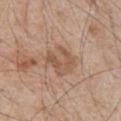No biopsy was performed on this lesion — it was imaged during a full skin examination and was not determined to be concerning. Captured under white-light illumination. The lesion-visualizer software estimated a lesion-to-skin contrast of about 6 (normalized; higher = more distinct). A close-up tile cropped from a whole-body skin photograph, about 15 mm across. Located on the chest. A male subject, in their mid- to late 60s.